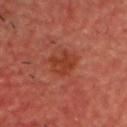notes: imaged on a skin check; not biopsied | illumination: cross-polarized | subject: male, aged 58 to 62 | image: 15 mm crop, total-body photography | site: the head or neck.